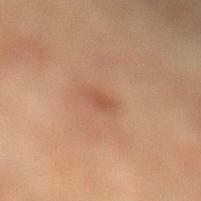The lesion was tiled from a total-body skin photograph and was not biopsied.